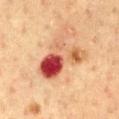<tbp_lesion>
<biopsy_status>not biopsied; imaged during a skin examination</biopsy_status>
<image>
  <source>total-body photography crop</source>
  <field_of_view_mm>15</field_of_view_mm>
</image>
<site>chest</site>
<patient>
  <sex>male</sex>
  <age_approx>60</age_approx>
</patient>
</tbp_lesion>